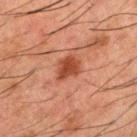Q: Was this lesion biopsied?
A: total-body-photography surveillance lesion; no biopsy
Q: Lesion location?
A: the upper back
Q: How was this image acquired?
A: ~15 mm tile from a whole-body skin photo
Q: Patient demographics?
A: male, about 50 years old
Q: What is the lesion's diameter?
A: about 3.5 mm